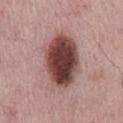The lesion was photographed on a routine skin check and not biopsied; there is no pathology result. The tile uses white-light illumination. The lesion is located on the mid back. Cropped from a whole-body photographic skin survey; the tile spans about 15 mm. Measured at roughly 7 mm in maximum diameter. The subject is a male about 65 years old.